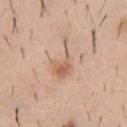Assessment: Part of a total-body skin-imaging series; this lesion was reviewed on a skin check and was not flagged for biopsy. Clinical summary: This is a white-light tile. A male patient, roughly 40 years of age. Automated image analysis of the tile measured an average lesion color of about L≈62 a*≈19 b*≈31 (CIELAB), a lesion–skin lightness drop of about 9, and a normalized lesion–skin contrast near 6. The analysis additionally found a border-irregularity index near 7/10, a color-variation rating of about 3.5/10, and peripheral color asymmetry of about 1. And it measured a nevus-likeness score of about 0/100 and a detector confidence of about 100 out of 100 that the crop contains a lesion. On the chest. Cropped from a whole-body photographic skin survey; the tile spans about 15 mm.